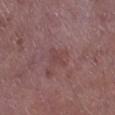Q: Is there a histopathology result?
A: imaged on a skin check; not biopsied
Q: What is the anatomic site?
A: the right lower leg
Q: How was the tile lit?
A: white-light
Q: What kind of image is this?
A: ~15 mm crop, total-body skin-cancer survey
Q: Patient demographics?
A: male, aged 68 to 72